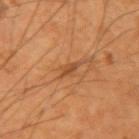The subject is a male approximately 55 years of age.
Automated image analysis of the tile measured a lesion color around L≈44 a*≈22 b*≈36 in CIELAB. And it measured a border-irregularity rating of about 4/10, a within-lesion color-variation index near 0/10, and radial color variation of about 0.
The recorded lesion diameter is about 2.5 mm.
This image is a 15 mm lesion crop taken from a total-body photograph.
Captured under cross-polarized illumination.
The lesion is located on the right upper arm.